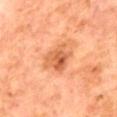Clinical impression: The lesion was photographed on a routine skin check and not biopsied; there is no pathology result. Clinical summary: The lesion-visualizer software estimated a lesion area of about 9.5 mm², an eccentricity of roughly 0.75, and a symmetry-axis asymmetry near 0.35. It also reported a border-irregularity rating of about 4/10, a color-variation rating of about 8/10, and a peripheral color-asymmetry measure near 3. From the back. The recorded lesion diameter is about 4.5 mm. A male subject, in their 70s. This is a cross-polarized tile. Cropped from a whole-body photographic skin survey; the tile spans about 15 mm.